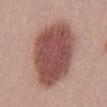Q: What lighting was used for the tile?
A: white-light illumination
Q: Who is the patient?
A: male, roughly 30 years of age
Q: What is the lesion's diameter?
A: about 11 mm
Q: What kind of image is this?
A: total-body-photography crop, ~15 mm field of view